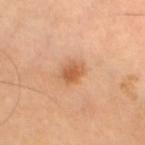Clinical impression: Captured during whole-body skin photography for melanoma surveillance; the lesion was not biopsied. Image and clinical context: Captured under cross-polarized illumination. Automated tile analysis of the lesion measured a footprint of about 4.5 mm² and a shape eccentricity near 0.5. And it measured a lesion color around L≈60 a*≈25 b*≈39 in CIELAB, a lesion–skin lightness drop of about 11, and a lesion-to-skin contrast of about 7.5 (normalized; higher = more distinct). The software also gave lesion-presence confidence of about 100/100. The patient is a female about 70 years old. From the left thigh. Approximately 2.5 mm at its widest. A 15 mm close-up tile from a total-body photography series done for melanoma screening.